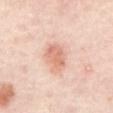follow-up: no biopsy performed (imaged during a skin exam) | lighting: cross-polarized illumination | size: ~3.5 mm (longest diameter) | patient: aged approximately 55 | imaging modality: 15 mm crop, total-body photography | anatomic site: the abdomen.